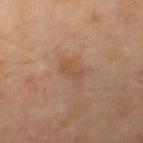  site: right thigh
  lesion_size:
    long_diameter_mm_approx: 2.5
  image:
    source: total-body photography crop
    field_of_view_mm: 15
  automated_metrics:
    area_mm2_approx: 3.0
    eccentricity: 0.9
    shape_asymmetry: 0.3
    border_irregularity_0_10: 3.5
    color_variation_0_10: 0.0
    peripheral_color_asymmetry: 0.0
    nevus_likeness_0_100: 0
    lesion_detection_confidence_0_100: 100
  patient:
    sex: male
    age_approx: 65
  lighting: cross-polarized